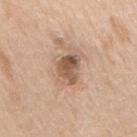| field | value |
|---|---|
| follow-up | imaged on a skin check; not biopsied |
| lesion diameter | about 4.5 mm |
| acquisition | ~15 mm crop, total-body skin-cancer survey |
| body site | the mid back |
| subject | male, approximately 65 years of age |